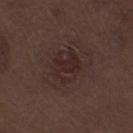No biopsy was performed on this lesion — it was imaged during a full skin examination and was not determined to be concerning. The patient is a male aged 68 to 72. Located on the right thigh. The lesion-visualizer software estimated an average lesion color of about L≈25 a*≈16 b*≈16 (CIELAB), roughly 5 lightness units darker than nearby skin, and a lesion-to-skin contrast of about 6 (normalized; higher = more distinct). Imaged with white-light lighting. Measured at roughly 4.5 mm in maximum diameter. Cropped from a whole-body photographic skin survey; the tile spans about 15 mm.